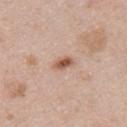workup = imaged on a skin check; not biopsied
body site = the upper back
lighting = white-light illumination
subject = male, in their 40s
image source = 15 mm crop, total-body photography